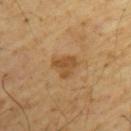Recorded during total-body skin imaging; not selected for excision or biopsy.
The lesion's longest dimension is about 3 mm.
A male subject aged 63 to 67.
This image is a 15 mm lesion crop taken from a total-body photograph.
The lesion is on the upper back.
This is a cross-polarized tile.